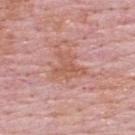Clinical summary:
A male patient in their mid-60s. A 15 mm close-up extracted from a 3D total-body photography capture. This is a white-light tile. Measured at roughly 4 mm in maximum diameter. An algorithmic analysis of the crop reported a footprint of about 10 mm² and an eccentricity of roughly 0.5. The analysis additionally found an average lesion color of about L≈58 a*≈24 b*≈29 (CIELAB), roughly 7 lightness units darker than nearby skin, and a normalized lesion–skin contrast near 6. The software also gave a within-lesion color-variation index near 3/10 and peripheral color asymmetry of about 1. And it measured an automated nevus-likeness rating near 0 out of 100 and lesion-presence confidence of about 100/100. Located on the upper back.